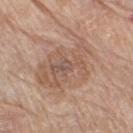{
  "biopsy_status": "not biopsied; imaged during a skin examination",
  "site": "left thigh",
  "lighting": "white-light",
  "lesion_size": {
    "long_diameter_mm_approx": 7.5
  },
  "patient": {
    "sex": "female",
    "age_approx": 75
  },
  "image": {
    "source": "total-body photography crop",
    "field_of_view_mm": 15
  },
  "automated_metrics": {
    "area_mm2_approx": 31.0,
    "eccentricity": 0.7,
    "shape_asymmetry": 0.25,
    "cielab_L": 57,
    "cielab_a": 18,
    "cielab_b": 28,
    "vs_skin_darker_L": 8.0,
    "vs_skin_contrast_norm": 5.5,
    "border_irregularity_0_10": 4.5,
    "color_variation_0_10": 5.0,
    "peripheral_color_asymmetry": 1.5
  }
}